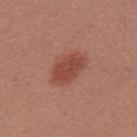The lesion is located on the upper back. A 15 mm close-up extracted from a 3D total-body photography capture. A female subject, roughly 25 years of age.